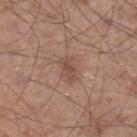Q: Lesion size?
A: ~3 mm (longest diameter)
Q: What is the imaging modality?
A: 15 mm crop, total-body photography
Q: Patient demographics?
A: male, aged approximately 60
Q: What did automated image analysis measure?
A: a footprint of about 4 mm², a shape eccentricity near 0.85, and a symmetry-axis asymmetry near 0.3; about 8 CIELAB-L* units darker than the surrounding skin and a normalized border contrast of about 6; a border-irregularity index near 3/10, a within-lesion color-variation index near 2/10, and peripheral color asymmetry of about 0.5
Q: What is the anatomic site?
A: the right lower leg